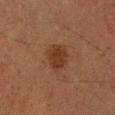| key | value |
|---|---|
| notes | total-body-photography surveillance lesion; no biopsy |
| location | the right upper arm |
| patient | male, aged approximately 75 |
| lesion size | about 3.5 mm |
| tile lighting | cross-polarized |
| image | total-body-photography crop, ~15 mm field of view |